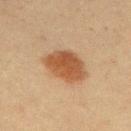The lesion was tiled from a total-body skin photograph and was not biopsied. The tile uses cross-polarized illumination. Located on the right upper arm. The patient is a male approximately 60 years of age. This image is a 15 mm lesion crop taken from a total-body photograph. The lesion's longest dimension is about 5 mm.